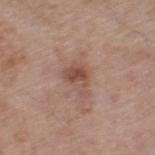<case>
  <biopsy_status>not biopsied; imaged during a skin examination</biopsy_status>
  <lesion_size>
    <long_diameter_mm_approx>3.5</long_diameter_mm_approx>
  </lesion_size>
  <site>leg</site>
  <patient>
    <sex>male</sex>
    <age_approx>80</age_approx>
  </patient>
  <lighting>white-light</lighting>
  <image>
    <source>total-body photography crop</source>
    <field_of_view_mm>15</field_of_view_mm>
  </image>
</case>